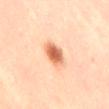The lesion was photographed on a routine skin check and not biopsied; there is no pathology result.
An algorithmic analysis of the crop reported a footprint of about 6.5 mm². And it measured a nevus-likeness score of about 100/100 and a detector confidence of about 100 out of 100 that the crop contains a lesion.
The subject is a female approximately 45 years of age.
The lesion is on the lower back.
Imaged with cross-polarized lighting.
A lesion tile, about 15 mm wide, cut from a 3D total-body photograph.